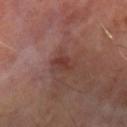illumination = cross-polarized
subject = male, in their mid- to late 60s
location = the left forearm
lesion size = ≈3 mm
image = total-body-photography crop, ~15 mm field of view